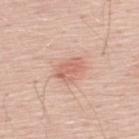Case summary:
- workup — imaged on a skin check; not biopsied
- patient — male, aged 48–52
- anatomic site — the upper back
- acquisition — total-body-photography crop, ~15 mm field of view